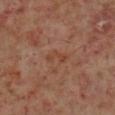The lesion was tiled from a total-body skin photograph and was not biopsied. A roughly 15 mm field-of-view crop from a total-body skin photograph. Longest diameter approximately 3 mm. Located on the leg. Imaged with cross-polarized lighting. The patient is a male aged approximately 60.